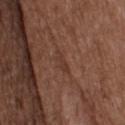Q: Is there a histopathology result?
A: imaged on a skin check; not biopsied
Q: Lesion size?
A: ~3 mm (longest diameter)
Q: Who is the patient?
A: male, about 50 years old
Q: What lighting was used for the tile?
A: white-light
Q: What is the anatomic site?
A: the head or neck
Q: What is the imaging modality?
A: total-body-photography crop, ~15 mm field of view